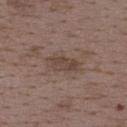Case summary:
– follow-up · imaged on a skin check; not biopsied
– lighting · white-light
– image · total-body-photography crop, ~15 mm field of view
– automated metrics · an automated nevus-likeness rating near 0 out of 100 and lesion-presence confidence of about 100/100
– lesion size · ~4.5 mm (longest diameter)
– subject · female, in their mid- to late 30s
– site · the upper back